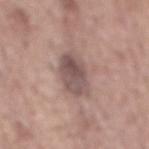Q: Was a biopsy performed?
A: catalogued during a skin exam; not biopsied
Q: What is the anatomic site?
A: the back
Q: Patient demographics?
A: male, aged 53–57
Q: How was the tile lit?
A: white-light
Q: What is the imaging modality?
A: 15 mm crop, total-body photography
Q: How large is the lesion?
A: ≈5.5 mm